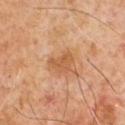Part of a total-body skin-imaging series; this lesion was reviewed on a skin check and was not flagged for biopsy. About 3 mm across. The lesion-visualizer software estimated an eccentricity of roughly 0.55. And it measured a mean CIELAB color near L≈55 a*≈23 b*≈38 and a normalized lesion–skin contrast near 6.5. The software also gave a border-irregularity index near 4/10, internal color variation of about 3 on a 0–10 scale, and a peripheral color-asymmetry measure near 1. A 15 mm crop from a total-body photograph taken for skin-cancer surveillance. A male patient aged 63 to 67. The tile uses cross-polarized illumination.